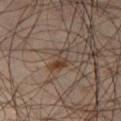{"biopsy_status": "not biopsied; imaged during a skin examination", "image": {"source": "total-body photography crop", "field_of_view_mm": 15}, "site": "leg", "lesion_size": {"long_diameter_mm_approx": 3.5}, "patient": {"sex": "male", "age_approx": 45}, "lighting": "cross-polarized"}